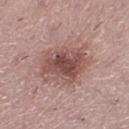Imaged during a routine full-body skin examination; the lesion was not biopsied and no histopathology is available.
The recorded lesion diameter is about 6.5 mm.
A close-up tile cropped from a whole-body skin photograph, about 15 mm across.
The lesion is located on the leg.
The lesion-visualizer software estimated a lesion area of about 21 mm², a shape eccentricity near 0.7, and two-axis asymmetry of about 0.25. And it measured a border-irregularity rating of about 3.5/10, a within-lesion color-variation index near 5.5/10, and peripheral color asymmetry of about 1.5.
The tile uses white-light illumination.
A female patient aged approximately 40.